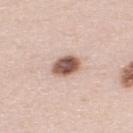This lesion was catalogued during total-body skin photography and was not selected for biopsy.
Automated image analysis of the tile measured a footprint of about 7 mm² and an outline eccentricity of about 0.6 (0 = round, 1 = elongated). And it measured an average lesion color of about L≈56 a*≈20 b*≈25 (CIELAB) and a lesion-to-skin contrast of about 12 (normalized; higher = more distinct). The software also gave border irregularity of about 1 on a 0–10 scale, a within-lesion color-variation index near 5.5/10, and a peripheral color-asymmetry measure near 1.5. The software also gave a nevus-likeness score of about 85/100.
The lesion is located on the upper back.
The recorded lesion diameter is about 3 mm.
Cropped from a total-body skin-imaging series; the visible field is about 15 mm.
A male patient aged approximately 35.
Imaged with white-light lighting.